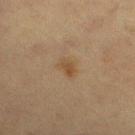The lesion was photographed on a routine skin check and not biopsied; there is no pathology result. A female subject in their mid- to late 50s. Imaged with cross-polarized lighting. Measured at roughly 2.5 mm in maximum diameter. From the leg. A 15 mm crop from a total-body photograph taken for skin-cancer surveillance.